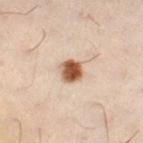Q: Lesion size?
A: ≈3 mm
Q: Lesion location?
A: the left leg
Q: What lighting was used for the tile?
A: cross-polarized
Q: What kind of image is this?
A: total-body-photography crop, ~15 mm field of view
Q: Patient demographics?
A: female, aged 28 to 32